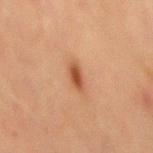Q: Is there a histopathology result?
A: no biopsy performed (imaged during a skin exam)
Q: Where on the body is the lesion?
A: the abdomen
Q: What kind of image is this?
A: 15 mm crop, total-body photography
Q: What are the patient's age and sex?
A: male, aged 68 to 72